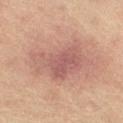{"biopsy_status": "not biopsied; imaged during a skin examination", "image": {"source": "total-body photography crop", "field_of_view_mm": 15}, "automated_metrics": {"area_mm2_approx": 21.0, "eccentricity": 0.7, "shape_asymmetry": 0.35, "border_irregularity_0_10": 5.0, "color_variation_0_10": 4.0, "peripheral_color_asymmetry": 1.0}, "patient": {"sex": "female", "age_approx": 65}, "site": "right thigh"}